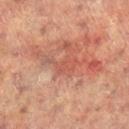Part of a total-body skin-imaging series; this lesion was reviewed on a skin check and was not flagged for biopsy. A female patient, about 80 years old. Cropped from a whole-body photographic skin survey; the tile spans about 15 mm. Automated image analysis of the tile measured a lesion color around L≈49 a*≈26 b*≈28 in CIELAB, roughly 7 lightness units darker than nearby skin, and a normalized lesion–skin contrast near 5. And it measured a border-irregularity index near 8/10, a within-lesion color-variation index near 1.5/10, and radial color variation of about 0.5. The lesion's longest dimension is about 4.5 mm. Located on the left leg.